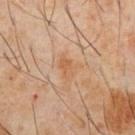Findings:
– biopsy status — total-body-photography surveillance lesion; no biopsy
– subject — male, aged 58–62
– lesion diameter — ≈3 mm
– body site — the abdomen
– image source — total-body-photography crop, ~15 mm field of view
– illumination — cross-polarized illumination
– automated metrics — a mean CIELAB color near L≈55 a*≈20 b*≈34, a lesion–skin lightness drop of about 5, and a lesion-to-skin contrast of about 5 (normalized; higher = more distinct); a border-irregularity rating of about 3/10, a color-variation rating of about 3/10, and radial color variation of about 1; an automated nevus-likeness rating near 0 out of 100 and a detector confidence of about 100 out of 100 that the crop contains a lesion